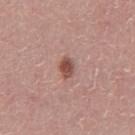The lesion was tiled from a total-body skin photograph and was not biopsied.
Imaged with white-light lighting.
Longest diameter approximately 2.5 mm.
From the abdomen.
A male patient, aged 43–47.
A 15 mm close-up tile from a total-body photography series done for melanoma screening.
Automated tile analysis of the lesion measured an average lesion color of about L≈50 a*≈23 b*≈25 (CIELAB), a lesion–skin lightness drop of about 12, and a normalized lesion–skin contrast near 9.